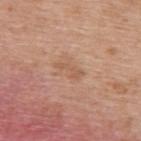Recorded during total-body skin imaging; not selected for excision or biopsy. A female subject, in their 40s. On the upper back. A close-up tile cropped from a whole-body skin photograph, about 15 mm across. The tile uses white-light illumination. Automated image analysis of the tile measured a footprint of about 2.5 mm², an eccentricity of roughly 0.95, and a shape-asymmetry score of about 0.5 (0 = symmetric). And it measured a mean CIELAB color near L≈57 a*≈21 b*≈32, roughly 7 lightness units darker than nearby skin, and a normalized lesion–skin contrast near 5.5. The software also gave a border-irregularity rating of about 7/10, a within-lesion color-variation index near 0/10, and a peripheral color-asymmetry measure near 0. Measured at roughly 3 mm in maximum diameter.